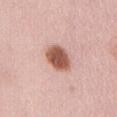follow-up: catalogued during a skin exam; not biopsied | subject: female, aged 48 to 52 | location: the front of the torso | image: total-body-photography crop, ~15 mm field of view | lesion size: ≈3.5 mm.